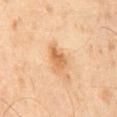Q: Was this lesion biopsied?
A: total-body-photography surveillance lesion; no biopsy
Q: What is the imaging modality?
A: 15 mm crop, total-body photography
Q: What is the anatomic site?
A: the mid back
Q: How large is the lesion?
A: about 3 mm
Q: What lighting was used for the tile?
A: cross-polarized illumination
Q: What are the patient's age and sex?
A: male, in their mid- to late 40s